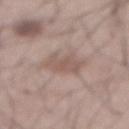| key | value |
|---|---|
| follow-up | imaged on a skin check; not biopsied |
| illumination | white-light |
| image | ~15 mm crop, total-body skin-cancer survey |
| patient | male, aged approximately 55 |
| TBP lesion metrics | an outline eccentricity of about 0.7 (0 = round, 1 = elongated) and a symmetry-axis asymmetry near 0.3; a lesion color around L≈54 a*≈17 b*≈23 in CIELAB, a lesion–skin lightness drop of about 8, and a normalized border contrast of about 5.5; a nevus-likeness score of about 0/100 and a detector confidence of about 100 out of 100 that the crop contains a lesion |
| location | the abdomen |